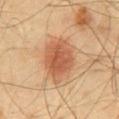The lesion was tiled from a total-body skin photograph and was not biopsied. A lesion tile, about 15 mm wide, cut from a 3D total-body photograph. A male patient, about 40 years old. Located on the back. Captured under cross-polarized illumination. The lesion's longest dimension is about 5 mm. Automated image analysis of the tile measured roughly 12 lightness units darker than nearby skin. The software also gave a border-irregularity index near 2/10, a color-variation rating of about 4/10, and a peripheral color-asymmetry measure near 1. The analysis additionally found a classifier nevus-likeness of about 100/100 and a lesion-detection confidence of about 100/100.